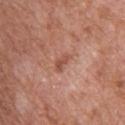notes: catalogued during a skin exam; not biopsied
body site: the upper back
subject: female, in their mid- to late 70s
tile lighting: white-light
image source: 15 mm crop, total-body photography
diameter: ≈2.5 mm